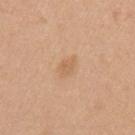The total-body-photography lesion software estimated an outline eccentricity of about 0.75 (0 = round, 1 = elongated) and a shape-asymmetry score of about 0.3 (0 = symmetric). And it measured a lesion–skin lightness drop of about 7 and a lesion-to-skin contrast of about 5 (normalized; higher = more distinct). The analysis additionally found a border-irregularity index near 3/10, a within-lesion color-variation index near 1/10, and a peripheral color-asymmetry measure near 0.5. On the left upper arm. Measured at roughly 2.5 mm in maximum diameter. Captured under white-light illumination. A female patient, aged 38 to 42. A 15 mm close-up tile from a total-body photography series done for melanoma screening.